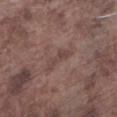<lesion>
  <biopsy_status>not biopsied; imaged during a skin examination</biopsy_status>
  <patient>
    <sex>male</sex>
    <age_approx>75</age_approx>
  </patient>
  <image>
    <source>total-body photography crop</source>
    <field_of_view_mm>15</field_of_view_mm>
  </image>
  <site>left lower leg</site>
  <lighting>white-light</lighting>
  <lesion_size>
    <long_diameter_mm_approx>3.5</long_diameter_mm_approx>
  </lesion_size>
</lesion>